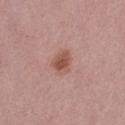Clinical impression:
Part of a total-body skin-imaging series; this lesion was reviewed on a skin check and was not flagged for biopsy.
Image and clinical context:
On the left thigh. Longest diameter approximately 3 mm. The subject is a female in their mid- to late 40s. The tile uses white-light illumination. Automated tile analysis of the lesion measured a lesion color around L≈53 a*≈23 b*≈26 in CIELAB, roughly 10 lightness units darker than nearby skin, and a normalized border contrast of about 7.5. And it measured a border-irregularity index near 1.5/10 and a color-variation rating of about 3/10. The software also gave an automated nevus-likeness rating near 85 out of 100 and lesion-presence confidence of about 100/100. A region of skin cropped from a whole-body photographic capture, roughly 15 mm wide.